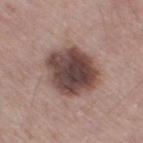Recorded during total-body skin imaging; not selected for excision or biopsy. From the right thigh. A lesion tile, about 15 mm wide, cut from a 3D total-body photograph. Imaged with white-light lighting. Measured at roughly 6 mm in maximum diameter. A male subject about 55 years old.